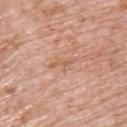The lesion was photographed on a routine skin check and not biopsied; there is no pathology result.
A male subject aged around 60.
From the upper back.
A 15 mm close-up extracted from a 3D total-body photography capture.